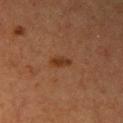| key | value |
|---|---|
| workup | catalogued during a skin exam; not biopsied |
| body site | the arm |
| lighting | cross-polarized |
| lesion diameter | ≈3 mm |
| subject | female, approximately 40 years of age |
| imaging modality | ~15 mm crop, total-body skin-cancer survey |